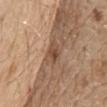Assessment:
This lesion was catalogued during total-body skin photography and was not selected for biopsy.
Context:
Measured at roughly 3 mm in maximum diameter. The lesion is on the chest. The subject is a male roughly 70 years of age. Cropped from a whole-body photographic skin survey; the tile spans about 15 mm.